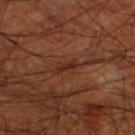{
  "lighting": "cross-polarized",
  "site": "left lower leg",
  "image": {
    "source": "total-body photography crop",
    "field_of_view_mm": 15
  },
  "patient": {
    "sex": "male",
    "age_approx": 70
  },
  "lesion_size": {
    "long_diameter_mm_approx": 3.0
  }
}